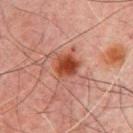notes = no biopsy performed (imaged during a skin exam); image source = 15 mm crop, total-body photography; diameter = ~3 mm (longest diameter); site = the mid back; tile lighting = cross-polarized; subject = male, aged 68–72.